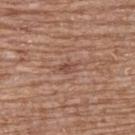Part of a total-body skin-imaging series; this lesion was reviewed on a skin check and was not flagged for biopsy.
A female subject aged approximately 80.
A 15 mm close-up tile from a total-body photography series done for melanoma screening.
The lesion is located on the upper back.